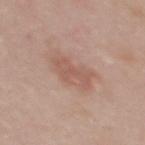Assessment: Part of a total-body skin-imaging series; this lesion was reviewed on a skin check and was not flagged for biopsy. Clinical summary: The lesion is located on the upper back. This is a white-light tile. Automated image analysis of the tile measured an area of roughly 9.5 mm², a shape eccentricity near 0.8, and a symmetry-axis asymmetry near 0.25. It also reported a within-lesion color-variation index near 2/10 and radial color variation of about 0.5. It also reported a nevus-likeness score of about 10/100. A female subject aged 38–42. About 4.5 mm across. A close-up tile cropped from a whole-body skin photograph, about 15 mm across.